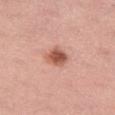patient:
  sex: female
  age_approx: 45
site: left thigh
lesion_size:
  long_diameter_mm_approx: 2.5
image:
  source: total-body photography crop
  field_of_view_mm: 15
lighting: white-light